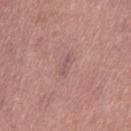Impression: Part of a total-body skin-imaging series; this lesion was reviewed on a skin check and was not flagged for biopsy.